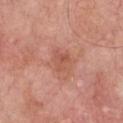Assessment:
Recorded during total-body skin imaging; not selected for excision or biopsy.
Background:
The lesion is on the chest. A male patient, roughly 60 years of age. A 15 mm close-up tile from a total-body photography series done for melanoma screening.